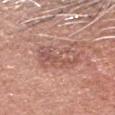notes = imaged on a skin check; not biopsied
lesion diameter = about 5 mm
tile lighting = white-light
patient = male, in their mid- to late 60s
image source = 15 mm crop, total-body photography
body site = the head or neck